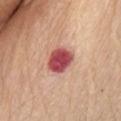Assessment:
No biopsy was performed on this lesion — it was imaged during a full skin examination and was not determined to be concerning.
Acquisition and patient details:
The lesion-visualizer software estimated a lesion area of about 8.5 mm², an eccentricity of roughly 0.55, and a shape-asymmetry score of about 0.1 (0 = symmetric). The analysis additionally found a border-irregularity rating of about 1/10, a color-variation rating of about 4/10, and radial color variation of about 1. The software also gave an automated nevus-likeness rating near 0 out of 100. A 15 mm close-up extracted from a 3D total-body photography capture. The lesion is on the abdomen. A female subject roughly 70 years of age. This is a white-light tile.